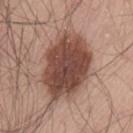<tbp_lesion>
  <biopsy_status>not biopsied; imaged during a skin examination</biopsy_status>
  <site>lower back</site>
  <lighting>white-light</lighting>
  <lesion_size>
    <long_diameter_mm_approx>9.0</long_diameter_mm_approx>
  </lesion_size>
  <image>
    <source>total-body photography crop</source>
    <field_of_view_mm>15</field_of_view_mm>
  </image>
  <automated_metrics>
    <peripheral_color_asymmetry>1.5</peripheral_color_asymmetry>
  </automated_metrics>
  <patient>
    <sex>male</sex>
    <age_approx>70</age_approx>
  </patient>
</tbp_lesion>